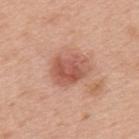– imaging modality — 15 mm crop, total-body photography
– anatomic site — the mid back
– lesion size — ≈4.5 mm
– patient — female, roughly 45 years of age
– lighting — white-light